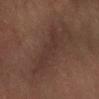{"biopsy_status": "not biopsied; imaged during a skin examination", "lesion_size": {"long_diameter_mm_approx": 5.5}, "lighting": "cross-polarized", "patient": {"sex": "male", "age_approx": 65}, "image": {"source": "total-body photography crop", "field_of_view_mm": 15}, "site": "left forearm"}